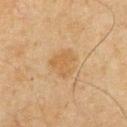| field | value |
|---|---|
| biopsy status | catalogued during a skin exam; not biopsied |
| image | ~15 mm crop, total-body skin-cancer survey |
| subject | male, approximately 65 years of age |
| body site | the chest |
| tile lighting | cross-polarized illumination |